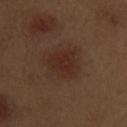Q: What are the patient's age and sex?
A: male, aged approximately 70
Q: What is the lesion's diameter?
A: about 4 mm
Q: Lesion location?
A: the left upper arm
Q: How was this image acquired?
A: ~15 mm crop, total-body skin-cancer survey